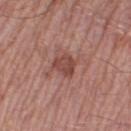Recorded during total-body skin imaging; not selected for excision or biopsy.
The tile uses white-light illumination.
A male patient, approximately 55 years of age.
Approximately 2.5 mm at its widest.
A 15 mm close-up extracted from a 3D total-body photography capture.
From the right thigh.